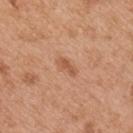Findings:
• location: the right upper arm
• acquisition: ~15 mm tile from a whole-body skin photo
• illumination: white-light illumination
• lesion size: about 3 mm
• patient: male, aged 53–57